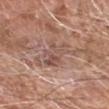Impression:
No biopsy was performed on this lesion — it was imaged during a full skin examination and was not determined to be concerning.
Acquisition and patient details:
Approximately 5 mm at its widest. This is a white-light tile. The lesion-visualizer software estimated a footprint of about 9 mm², a shape eccentricity near 0.85, and a symmetry-axis asymmetry near 0.35. The analysis additionally found a peripheral color-asymmetry measure near 2. The software also gave a nevus-likeness score of about 0/100 and a detector confidence of about 90 out of 100 that the crop contains a lesion. The lesion is located on the right forearm. A male subject, in their mid-70s. Cropped from a total-body skin-imaging series; the visible field is about 15 mm.